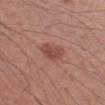notes = no biopsy performed (imaged during a skin exam)
location = the right forearm
automated lesion analysis = an average lesion color of about L≈48 a*≈23 b*≈24 (CIELAB), roughly 9 lightness units darker than nearby skin, and a normalized border contrast of about 6.5; a classifier nevus-likeness of about 50/100 and a lesion-detection confidence of about 100/100
subject = male, aged around 55
imaging modality = ~15 mm tile from a whole-body skin photo
size = about 3 mm
lighting = white-light